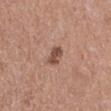Clinical impression:
The lesion was tiled from a total-body skin photograph and was not biopsied.
Clinical summary:
Cropped from a whole-body photographic skin survey; the tile spans about 15 mm. On the right lower leg. A female subject, aged 48 to 52. Approximately 2.5 mm at its widest.